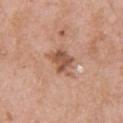Assessment: Recorded during total-body skin imaging; not selected for excision or biopsy. Background: A male patient aged 53–57. The lesion is located on the upper back. Longest diameter approximately 3.5 mm. A close-up tile cropped from a whole-body skin photograph, about 15 mm across. The tile uses white-light illumination.